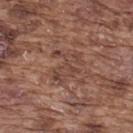The lesion was photographed on a routine skin check and not biopsied; there is no pathology result.
The lesion-visualizer software estimated an area of roughly 9 mm², an eccentricity of roughly 0.55, and a symmetry-axis asymmetry near 0.55. The software also gave a lesion color around L≈43 a*≈20 b*≈25 in CIELAB and a normalized border contrast of about 5.5. And it measured a border-irregularity rating of about 9/10, a color-variation rating of about 2.5/10, and a peripheral color-asymmetry measure near 0.5.
Captured under white-light illumination.
Located on the back.
The patient is a male aged approximately 75.
A close-up tile cropped from a whole-body skin photograph, about 15 mm across.
The lesion's longest dimension is about 4 mm.